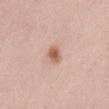Clinical impression:
The lesion was tiled from a total-body skin photograph and was not biopsied.
Acquisition and patient details:
On the lower back. A female patient, in their 30s. This image is a 15 mm lesion crop taken from a total-body photograph.